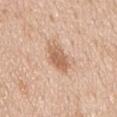Recorded during total-body skin imaging; not selected for excision or biopsy.
The patient is a male in their mid- to late 60s.
A 15 mm close-up extracted from a 3D total-body photography capture.
The tile uses white-light illumination.
On the chest.
About 4 mm across.
The lesion-visualizer software estimated a lesion area of about 7 mm², an outline eccentricity of about 0.9 (0 = round, 1 = elongated), and a shape-asymmetry score of about 0.2 (0 = symmetric). And it measured a mean CIELAB color near L≈62 a*≈20 b*≈33 and roughly 12 lightness units darker than nearby skin.